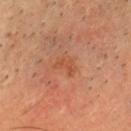biopsy status: catalogued during a skin exam; not biopsied | acquisition: 15 mm crop, total-body photography | patient: male, in their mid- to late 40s | site: the head or neck.